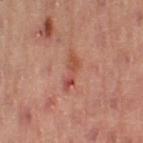Imaged during a routine full-body skin examination; the lesion was not biopsied and no histopathology is available. Located on the left thigh. A close-up tile cropped from a whole-body skin photograph, about 15 mm across. A female patient, approximately 55 years of age.